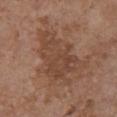The lesion was tiled from a total-body skin photograph and was not biopsied.
A female patient aged 63–67.
From the chest.
An algorithmic analysis of the crop reported an area of roughly 24 mm², an outline eccentricity of about 0.75 (0 = round, 1 = elongated), and a symmetry-axis asymmetry near 0.4.
The lesion's longest dimension is about 7.5 mm.
Cropped from a total-body skin-imaging series; the visible field is about 15 mm.